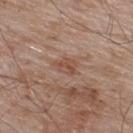biopsy_status: not biopsied; imaged during a skin examination